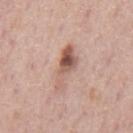Impression: Imaged during a routine full-body skin examination; the lesion was not biopsied and no histopathology is available. Background: Automated tile analysis of the lesion measured a border-irregularity index near 4.5/10, a color-variation rating of about 8.5/10, and peripheral color asymmetry of about 3. Cropped from a whole-body photographic skin survey; the tile spans about 15 mm. The subject is a male in their mid- to late 70s. The lesion's longest dimension is about 5.5 mm. Located on the back. The tile uses white-light illumination.